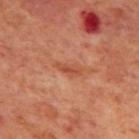The lesion was tiled from a total-body skin photograph and was not biopsied. The total-body-photography lesion software estimated a lesion color around L≈48 a*≈28 b*≈34 in CIELAB. The software also gave a color-variation rating of about 0.5/10 and a peripheral color-asymmetry measure near 0. A male subject aged approximately 70. A 15 mm close-up tile from a total-body photography series done for melanoma screening. From the mid back. Imaged with cross-polarized lighting.